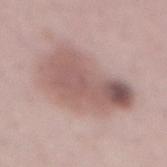Notes:
– subject: female, about 50 years old
– body site: the abdomen
– illumination: white-light illumination
– image source: 15 mm crop, total-body photography